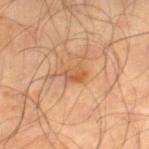Clinical impression: The lesion was tiled from a total-body skin photograph and was not biopsied. Background: Approximately 2.5 mm at its widest. This is a cross-polarized tile. Located on the leg. Cropped from a whole-body photographic skin survey; the tile spans about 15 mm. A male patient aged 63–67.